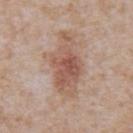Q: Was a biopsy performed?
A: catalogued during a skin exam; not biopsied
Q: What are the patient's age and sex?
A: male, aged 63 to 67
Q: What kind of image is this?
A: ~15 mm tile from a whole-body skin photo
Q: Lesion location?
A: the abdomen